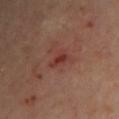biopsy_status: not biopsied; imaged during a skin examination
lesion_size:
  long_diameter_mm_approx: 2.5
site: left upper arm
image:
  source: total-body photography crop
  field_of_view_mm: 15
patient:
  sex: female
  age_approx: 60
lighting: cross-polarized